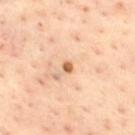biopsy_status: not biopsied; imaged during a skin examination
site: upper back
image:
  source: total-body photography crop
  field_of_view_mm: 15
patient:
  sex: male
  age_approx: 45